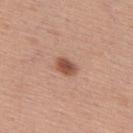Captured during whole-body skin photography for melanoma surveillance; the lesion was not biopsied.
The recorded lesion diameter is about 2.5 mm.
A 15 mm close-up extracted from a 3D total-body photography capture.
Captured under white-light illumination.
A male patient approximately 55 years of age.
On the upper back.
The total-body-photography lesion software estimated a shape eccentricity near 0.75. It also reported a color-variation rating of about 3/10. The software also gave an automated nevus-likeness rating near 100 out of 100 and a detector confidence of about 100 out of 100 that the crop contains a lesion.